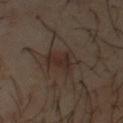The lesion was photographed on a routine skin check and not biopsied; there is no pathology result.